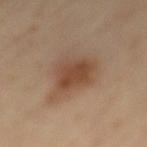notes: catalogued during a skin exam; not biopsied | automated lesion analysis: an area of roughly 16 mm², an eccentricity of roughly 0.6, and a shape-asymmetry score of about 0.35 (0 = symmetric); an average lesion color of about L≈47 a*≈18 b*≈29 (CIELAB) | site: the mid back | image source: total-body-photography crop, ~15 mm field of view | illumination: cross-polarized illumination | subject: male, approximately 65 years of age | lesion size: ~5.5 mm (longest diameter).